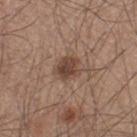Recorded during total-body skin imaging; not selected for excision or biopsy.
Captured under white-light illumination.
The patient is a male in their mid-40s.
The lesion's longest dimension is about 3 mm.
A lesion tile, about 15 mm wide, cut from a 3D total-body photograph.
The lesion is on the left thigh.